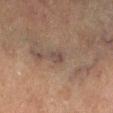Notes:
– workup: imaged on a skin check; not biopsied
– lesion size: ~2.5 mm (longest diameter)
– subject: female, aged 58 to 62
– site: the leg
– imaging modality: total-body-photography crop, ~15 mm field of view
– TBP lesion metrics: a lesion area of about 3.5 mm², an eccentricity of roughly 0.7, and a symmetry-axis asymmetry near 0.25; a lesion color around L≈37 a*≈12 b*≈18 in CIELAB and roughly 6 lightness units darker than nearby skin; a color-variation rating of about 2/10; a detector confidence of about 60 out of 100 that the crop contains a lesion